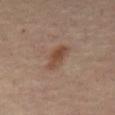This lesion was catalogued during total-body skin photography and was not selected for biopsy.
The recorded lesion diameter is about 4.5 mm.
Captured under cross-polarized illumination.
A subject approximately 55 years of age.
A close-up tile cropped from a whole-body skin photograph, about 15 mm across.
Located on the chest.